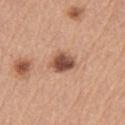Impression:
Captured during whole-body skin photography for melanoma surveillance; the lesion was not biopsied.
Clinical summary:
A 15 mm crop from a total-body photograph taken for skin-cancer surveillance. This is a white-light tile. About 3.5 mm across. A female patient aged approximately 65. The lesion is located on the left upper arm.